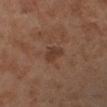Background: On the left lower leg. A close-up tile cropped from a whole-body skin photograph, about 15 mm across. A male patient approximately 70 years of age. The tile uses cross-polarized illumination. Approximately 2.5 mm at its widest.